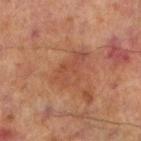notes=catalogued during a skin exam; not biopsied | patient=male, aged around 70 | site=the left lower leg | diameter=≈5 mm | tile lighting=cross-polarized | image=total-body-photography crop, ~15 mm field of view.